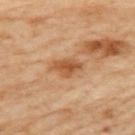Assessment:
Imaged during a routine full-body skin examination; the lesion was not biopsied and no histopathology is available.
Image and clinical context:
A 15 mm crop from a total-body photograph taken for skin-cancer surveillance. A female patient in their 60s. From the left upper arm. Measured at roughly 3.5 mm in maximum diameter.